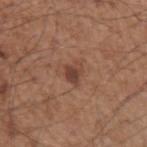Recorded during total-body skin imaging; not selected for excision or biopsy. This is a white-light tile. Measured at roughly 2.5 mm in maximum diameter. The total-body-photography lesion software estimated a footprint of about 4 mm², an outline eccentricity of about 0.55 (0 = round, 1 = elongated), and a symmetry-axis asymmetry near 0.4. The software also gave an automated nevus-likeness rating near 55 out of 100 and a detector confidence of about 100 out of 100 that the crop contains a lesion. A 15 mm crop from a total-body photograph taken for skin-cancer surveillance. The lesion is located on the left upper arm. A male patient, aged 53 to 57.